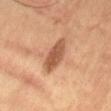Impression: Imaged during a routine full-body skin examination; the lesion was not biopsied and no histopathology is available. Context: Cropped from a total-body skin-imaging series; the visible field is about 15 mm. From the abdomen. The total-body-photography lesion software estimated a lesion area of about 9 mm² and a shape eccentricity near 0.9. The analysis additionally found border irregularity of about 2.5 on a 0–10 scale, a color-variation rating of about 3.5/10, and radial color variation of about 1.5. The software also gave a nevus-likeness score of about 5/100 and lesion-presence confidence of about 100/100. The lesion's longest dimension is about 5 mm. Imaged with cross-polarized lighting. A male subject, aged approximately 55.